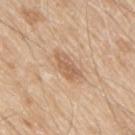Recorded during total-body skin imaging; not selected for excision or biopsy.
The subject is a male aged around 80.
The lesion is on the upper back.
A close-up tile cropped from a whole-body skin photograph, about 15 mm across.
Longest diameter approximately 4 mm.
Automated image analysis of the tile measured a shape eccentricity near 0.85 and a shape-asymmetry score of about 0.2 (0 = symmetric). It also reported a border-irregularity index near 2.5/10 and radial color variation of about 1. The analysis additionally found a nevus-likeness score of about 5/100 and a lesion-detection confidence of about 100/100.